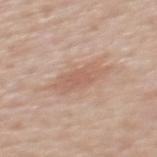Q: How was this image acquired?
A: total-body-photography crop, ~15 mm field of view
Q: Where on the body is the lesion?
A: the upper back
Q: What lighting was used for the tile?
A: white-light
Q: Patient demographics?
A: female, approximately 60 years of age
Q: What is the lesion's diameter?
A: ≈5.5 mm
Q: What did automated image analysis measure?
A: a lesion color around L≈60 a*≈20 b*≈28 in CIELAB, a lesion–skin lightness drop of about 8, and a normalized border contrast of about 5.5; border irregularity of about 3.5 on a 0–10 scale and a within-lesion color-variation index near 2/10; an automated nevus-likeness rating near 60 out of 100 and a detector confidence of about 100 out of 100 that the crop contains a lesion